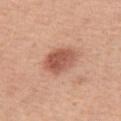• workup · catalogued during a skin exam; not biopsied
• lesion diameter · about 4 mm
• automated lesion analysis · an area of roughly 9.5 mm², an eccentricity of roughly 0.7, and two-axis asymmetry of about 0.15; a color-variation rating of about 4.5/10 and peripheral color asymmetry of about 1.5
• tile lighting · white-light
• acquisition · ~15 mm crop, total-body skin-cancer survey
• location · the right thigh
• patient · female, in their mid- to late 50s